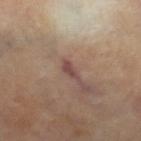Q: Is there a histopathology result?
A: total-body-photography surveillance lesion; no biopsy
Q: How was the tile lit?
A: cross-polarized
Q: Automated lesion metrics?
A: a border-irregularity rating of about 4/10, internal color variation of about 0 on a 0–10 scale, and radial color variation of about 0
Q: Where on the body is the lesion?
A: the right lower leg
Q: Lesion size?
A: ~2.5 mm (longest diameter)
Q: Who is the patient?
A: female, aged around 65
Q: What is the imaging modality?
A: total-body-photography crop, ~15 mm field of view